The patient is a male in their mid-60s; on the head or neck; this image is a 15 mm lesion crop taken from a total-body photograph.
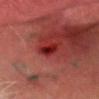{"lesion_size": {"long_diameter_mm_approx": 2.5}, "lighting": "cross-polarized", "diagnosis": {"histopathology": "nodular basal cell carcinoma", "malignancy": "malignant", "taxonomic_path": ["Malignant", "Malignant adnexal epithelial proliferations - Follicular", "Basal cell carcinoma", "Basal cell carcinoma, Nodular"]}}